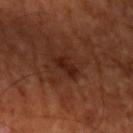Q: Was a biopsy performed?
A: imaged on a skin check; not biopsied
Q: What is the imaging modality?
A: 15 mm crop, total-body photography
Q: What is the anatomic site?
A: the left upper arm
Q: Illumination type?
A: cross-polarized
Q: Patient demographics?
A: approximately 65 years of age
Q: What is the lesion's diameter?
A: about 3 mm